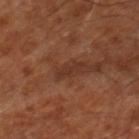A subject in their mid-60s.
The lesion-visualizer software estimated an area of roughly 3 mm², an outline eccentricity of about 0.95 (0 = round, 1 = elongated), and two-axis asymmetry of about 0.35. The software also gave a lesion color around L≈32 a*≈22 b*≈27 in CIELAB.
Located on the leg.
About 3 mm across.
Cropped from a total-body skin-imaging series; the visible field is about 15 mm.
This is a cross-polarized tile.